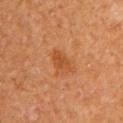follow-up=catalogued during a skin exam; not biopsied | patient=female | image source=total-body-photography crop, ~15 mm field of view | location=the right upper arm | automated lesion analysis=a lesion–skin lightness drop of about 8 and a normalized border contrast of about 6.5; an automated nevus-likeness rating near 25 out of 100 and lesion-presence confidence of about 100/100 | size=~2.5 mm (longest diameter).